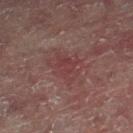Recorded during total-body skin imaging; not selected for excision or biopsy.
A male patient, aged approximately 60.
A 15 mm close-up extracted from a 3D total-body photography capture.
The lesion is located on the right lower leg.